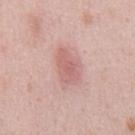This lesion was catalogued during total-body skin photography and was not selected for biopsy. The subject is a male roughly 40 years of age. On the abdomen. A 15 mm crop from a total-body photograph taken for skin-cancer surveillance. Approximately 4.5 mm at its widest.